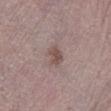Impression: Captured during whole-body skin photography for melanoma surveillance; the lesion was not biopsied. Background: About 2.5 mm across. The lesion-visualizer software estimated a classifier nevus-likeness of about 55/100 and a detector confidence of about 100 out of 100 that the crop contains a lesion. A female subject aged approximately 65. Captured under white-light illumination. The lesion is on the left lower leg. A roughly 15 mm field-of-view crop from a total-body skin photograph.